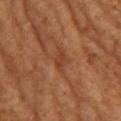Part of a total-body skin-imaging series; this lesion was reviewed on a skin check and was not flagged for biopsy. A lesion tile, about 15 mm wide, cut from a 3D total-body photograph. A female subject, aged 63 to 67. On the upper back. The total-body-photography lesion software estimated a footprint of about 3 mm², an eccentricity of roughly 0.85, and two-axis asymmetry of about 0.25. The software also gave an automated nevus-likeness rating near 0 out of 100 and a lesion-detection confidence of about 100/100. Measured at roughly 2.5 mm in maximum diameter.